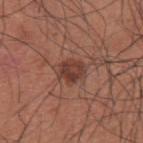Q: Was this lesion biopsied?
A: imaged on a skin check; not biopsied
Q: Lesion location?
A: the upper back
Q: Illumination type?
A: white-light
Q: How was this image acquired?
A: ~15 mm crop, total-body skin-cancer survey
Q: Automated lesion metrics?
A: an eccentricity of roughly 0.65 and two-axis asymmetry of about 0.2; a lesion-to-skin contrast of about 8 (normalized; higher = more distinct); a border-irregularity rating of about 2/10; a lesion-detection confidence of about 100/100
Q: What are the patient's age and sex?
A: male, in their mid- to late 30s